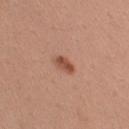<lesion>
  <biopsy_status>not biopsied; imaged during a skin examination</biopsy_status>
  <automated_metrics>
    <area_mm2_approx>3.5</area_mm2_approx>
    <eccentricity>0.8</eccentricity>
    <shape_asymmetry>0.35</shape_asymmetry>
    <border_irregularity_0_10>3.0</border_irregularity_0_10>
    <peripheral_color_asymmetry>0.5</peripheral_color_asymmetry>
    <nevus_likeness_0_100>95</nevus_likeness_0_100>
    <lesion_detection_confidence_0_100>100</lesion_detection_confidence_0_100>
  </automated_metrics>
  <patient>
    <sex>female</sex>
    <age_approx>30</age_approx>
  </patient>
  <image>
    <source>total-body photography crop</source>
    <field_of_view_mm>15</field_of_view_mm>
  </image>
  <site>left upper arm</site>
  <lesion_size>
    <long_diameter_mm_approx>2.5</long_diameter_mm_approx>
  </lesion_size>
  <lighting>white-light</lighting>
</lesion>